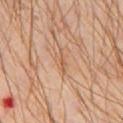Assessment: The lesion was photographed on a routine skin check and not biopsied; there is no pathology result. Clinical summary: On the chest. Automated tile analysis of the lesion measured a lesion color around L≈58 a*≈19 b*≈33 in CIELAB, roughly 6 lightness units darker than nearby skin, and a normalized lesion–skin contrast near 5. The software also gave a classifier nevus-likeness of about 0/100 and a lesion-detection confidence of about 65/100. Cropped from a total-body skin-imaging series; the visible field is about 15 mm. A male patient about 30 years old. Captured under cross-polarized illumination.